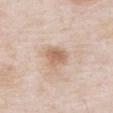Acquisition and patient details: The tile uses white-light illumination. The lesion's longest dimension is about 3 mm. The patient is a male in their mid- to late 50s. Cropped from a total-body skin-imaging series; the visible field is about 15 mm. Located on the chest.